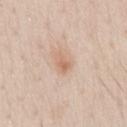The lesion was photographed on a routine skin check and not biopsied; there is no pathology result. The lesion is on the chest. A region of skin cropped from a whole-body photographic capture, roughly 15 mm wide. A male patient, roughly 30 years of age. Imaged with white-light lighting. The lesion's longest dimension is about 2.5 mm.